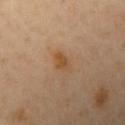Q: Was a biopsy performed?
A: no biopsy performed (imaged during a skin exam)
Q: Lesion location?
A: the left upper arm
Q: Patient demographics?
A: female, roughly 50 years of age
Q: How large is the lesion?
A: ≈3 mm
Q: What kind of image is this?
A: total-body-photography crop, ~15 mm field of view
Q: How was the tile lit?
A: cross-polarized illumination